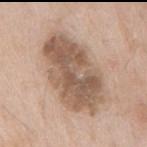Impression: The lesion was photographed on a routine skin check and not biopsied; there is no pathology result. Acquisition and patient details: A lesion tile, about 15 mm wide, cut from a 3D total-body photograph. The lesion is located on the mid back. A male subject aged approximately 65. Automated image analysis of the tile measured a normalized lesion–skin contrast near 9.